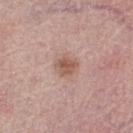Q: Was a biopsy performed?
A: total-body-photography surveillance lesion; no biopsy
Q: Who is the patient?
A: female, aged 63 to 67
Q: Automated lesion metrics?
A: a border-irregularity rating of about 2.5/10 and a peripheral color-asymmetry measure near 1; a detector confidence of about 100 out of 100 that the crop contains a lesion
Q: What kind of image is this?
A: ~15 mm tile from a whole-body skin photo
Q: What is the anatomic site?
A: the left thigh
Q: What is the lesion's diameter?
A: ~2.5 mm (longest diameter)